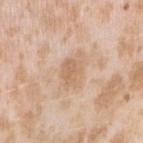| field | value |
|---|---|
| biopsy status | catalogued during a skin exam; not biopsied |
| acquisition | 15 mm crop, total-body photography |
| lesion size | ≈3 mm |
| patient | female, aged 23–27 |
| anatomic site | the arm |